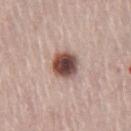Image and clinical context:
A male subject, in their mid-60s. Measured at roughly 3.5 mm in maximum diameter. This is a white-light tile. On the right thigh. Automated tile analysis of the lesion measured a lesion area of about 9 mm², an outline eccentricity of about 0.5 (0 = round, 1 = elongated), and a shape-asymmetry score of about 0.1 (0 = symmetric). A 15 mm close-up extracted from a 3D total-body photography capture.